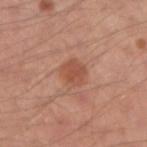| feature | finding |
|---|---|
| body site | the right upper arm |
| diameter | about 3 mm |
| tile lighting | white-light illumination |
| image | ~15 mm crop, total-body skin-cancer survey |
| subject | male, approximately 30 years of age |